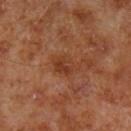Captured during whole-body skin photography for melanoma surveillance; the lesion was not biopsied. A male subject, approximately 70 years of age. Approximately 3.5 mm at its widest. The lesion is located on the leg. Cropped from a whole-body photographic skin survey; the tile spans about 15 mm. Imaged with cross-polarized lighting.